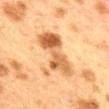Automated tile analysis of the lesion measured a classifier nevus-likeness of about 15/100 and lesion-presence confidence of about 100/100.
From the mid back.
A female subject, in their 40s.
Cropped from a whole-body photographic skin survey; the tile spans about 15 mm.
Imaged with cross-polarized lighting.
Longest diameter approximately 6.5 mm.